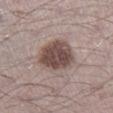Q: Was a biopsy performed?
A: total-body-photography surveillance lesion; no biopsy
Q: What is the lesion's diameter?
A: ≈4.5 mm
Q: What did automated image analysis measure?
A: a color-variation rating of about 4/10; an automated nevus-likeness rating near 20 out of 100 and lesion-presence confidence of about 100/100
Q: Patient demographics?
A: male, about 70 years old
Q: What kind of image is this?
A: ~15 mm crop, total-body skin-cancer survey
Q: Lesion location?
A: the right lower leg